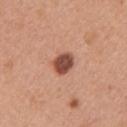anatomic site — the arm
diameter — ≈3 mm
subject — female, approximately 35 years of age
imaging modality — ~15 mm tile from a whole-body skin photo
TBP lesion metrics — a footprint of about 6 mm² and an outline eccentricity of about 0.55 (0 = round, 1 = elongated); an automated nevus-likeness rating near 95 out of 100
tile lighting — white-light illumination